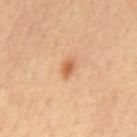Q: Is there a histopathology result?
A: total-body-photography surveillance lesion; no biopsy
Q: How was this image acquired?
A: ~15 mm crop, total-body skin-cancer survey
Q: How large is the lesion?
A: about 2 mm
Q: Patient demographics?
A: male, approximately 60 years of age
Q: Automated lesion metrics?
A: a lesion area of about 2.5 mm², an outline eccentricity of about 0.75 (0 = round, 1 = elongated), and two-axis asymmetry of about 0.25; an average lesion color of about L≈59 a*≈24 b*≈38 (CIELAB), about 11 CIELAB-L* units darker than the surrounding skin, and a normalized lesion–skin contrast near 7.5; internal color variation of about 2 on a 0–10 scale and peripheral color asymmetry of about 0.5; a nevus-likeness score of about 90/100 and lesion-presence confidence of about 100/100
Q: Lesion location?
A: the mid back